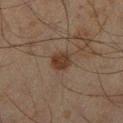– notes: no biopsy performed (imaged during a skin exam)
– subject: male, aged 43 to 47
– tile lighting: cross-polarized illumination
– imaging modality: 15 mm crop, total-body photography
– automated metrics: an automated nevus-likeness rating near 75 out of 100 and a lesion-detection confidence of about 100/100
– anatomic site: the leg